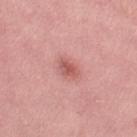No biopsy was performed on this lesion — it was imaged during a full skin examination and was not determined to be concerning.
Captured under white-light illumination.
The subject is a female about 40 years old.
The lesion is on the left thigh.
A 15 mm crop from a total-body photograph taken for skin-cancer surveillance.
The total-body-photography lesion software estimated a shape eccentricity near 0.8. It also reported a border-irregularity index near 3.5/10, a color-variation rating of about 2/10, and a peripheral color-asymmetry measure near 0.5.